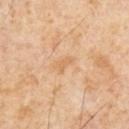Case summary:
* workup — no biopsy performed (imaged during a skin exam)
* lesion diameter — about 2.5 mm
* patient — male, approximately 65 years of age
* image-analysis metrics — a lesion color around L≈64 a*≈19 b*≈38 in CIELAB and roughly 6 lightness units darker than nearby skin; a border-irregularity rating of about 3.5/10; a classifier nevus-likeness of about 0/100 and a lesion-detection confidence of about 100/100
* image — ~15 mm crop, total-body skin-cancer survey